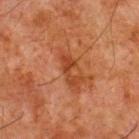A region of skin cropped from a whole-body photographic capture, roughly 15 mm wide.
The subject is a male approximately 65 years of age.
On the upper back.